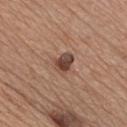Q: Patient demographics?
A: male, aged 63–67
Q: Lesion location?
A: the chest
Q: What is the imaging modality?
A: ~15 mm tile from a whole-body skin photo